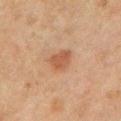• follow-up — catalogued during a skin exam; not biopsied
• illumination — cross-polarized
• imaging modality — 15 mm crop, total-body photography
• body site — the left upper arm
• subject — male, aged 48–52
• diameter — about 3 mm
• automated metrics — a lesion color around L≈43 a*≈20 b*≈29 in CIELAB